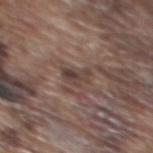Image and clinical context: A 15 mm close-up tile from a total-body photography series done for melanoma screening. The patient is a male in their mid-70s. Automated tile analysis of the lesion measured a lesion color around L≈41 a*≈15 b*≈20 in CIELAB, about 9 CIELAB-L* units darker than the surrounding skin, and a normalized lesion–skin contrast near 7.5. The analysis additionally found a border-irregularity rating of about 3.5/10, a color-variation rating of about 5.5/10, and radial color variation of about 2.5. On the upper back.